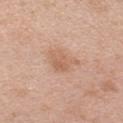<tbp_lesion>
<biopsy_status>not biopsied; imaged during a skin examination</biopsy_status>
<automated_metrics>
  <area_mm2_approx>6.0</area_mm2_approx>
  <eccentricity>0.65</eccentricity>
  <shape_asymmetry>0.35</shape_asymmetry>
  <cielab_L>61</cielab_L>
  <cielab_a>21</cielab_a>
  <cielab_b>32</cielab_b>
  <vs_skin_darker_L>8.0</vs_skin_darker_L>
  <vs_skin_contrast_norm>6.0</vs_skin_contrast_norm>
  <border_irregularity_0_10>4.0</border_irregularity_0_10>
  <color_variation_0_10>3.0</color_variation_0_10>
  <peripheral_color_asymmetry>1.0</peripheral_color_asymmetry>
  <nevus_likeness_0_100>5</nevus_likeness_0_100>
</automated_metrics>
<patient>
  <sex>female</sex>
  <age_approx>40</age_approx>
</patient>
<image>
  <source>total-body photography crop</source>
  <field_of_view_mm>15</field_of_view_mm>
</image>
<lesion_size>
  <long_diameter_mm_approx>3.5</long_diameter_mm_approx>
</lesion_size>
<lighting>white-light</lighting>
<site>left upper arm</site>
</tbp_lesion>